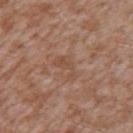Case summary:
* workup: no biopsy performed (imaged during a skin exam)
* imaging modality: 15 mm crop, total-body photography
* illumination: white-light illumination
* location: the left upper arm
* lesion size: about 3.5 mm
* patient: male, in their mid-40s
* automated metrics: a normalized border contrast of about 5; a nevus-likeness score of about 0/100 and a detector confidence of about 100 out of 100 that the crop contains a lesion